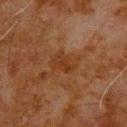Impression: Captured during whole-body skin photography for melanoma surveillance; the lesion was not biopsied. Image and clinical context: Captured under cross-polarized illumination. A 15 mm crop from a total-body photograph taken for skin-cancer surveillance. From the upper back. The total-body-photography lesion software estimated a lesion-detection confidence of about 100/100. A male subject, aged 78 to 82. About 3 mm across.